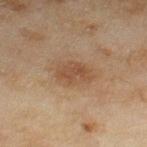Recorded during total-body skin imaging; not selected for excision or biopsy.
The patient is a female in their 60s.
The lesion-visualizer software estimated an eccentricity of roughly 0.8 and a symmetry-axis asymmetry near 0.2. And it measured a nevus-likeness score of about 55/100 and a lesion-detection confidence of about 100/100.
The recorded lesion diameter is about 4 mm.
From the right thigh.
Captured under cross-polarized illumination.
Cropped from a whole-body photographic skin survey; the tile spans about 15 mm.